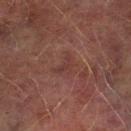Q: Is there a histopathology result?
A: catalogued during a skin exam; not biopsied
Q: What did automated image analysis measure?
A: a lesion area of about 3.5 mm², an eccentricity of roughly 0.85, and two-axis asymmetry of about 0.55; a nevus-likeness score of about 0/100 and lesion-presence confidence of about 95/100
Q: How was this image acquired?
A: total-body-photography crop, ~15 mm field of view
Q: Who is the patient?
A: male, in their mid-70s
Q: What is the anatomic site?
A: the left lower leg
Q: What is the lesion's diameter?
A: about 3 mm
Q: What lighting was used for the tile?
A: cross-polarized illumination